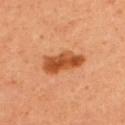<lesion>
  <biopsy_status>not biopsied; imaged during a skin examination</biopsy_status>
  <image>
    <source>total-body photography crop</source>
    <field_of_view_mm>15</field_of_view_mm>
  </image>
  <patient>
    <sex>female</sex>
    <age_approx>40</age_approx>
  </patient>
  <lesion_size>
    <long_diameter_mm_approx>5.0</long_diameter_mm_approx>
  </lesion_size>
  <site>upper back</site>
</lesion>